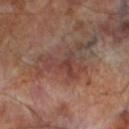No biopsy was performed on this lesion — it was imaged during a full skin examination and was not determined to be concerning. Captured under cross-polarized illumination. The patient is a male aged around 70. The lesion's longest dimension is about 4 mm. A roughly 15 mm field-of-view crop from a total-body skin photograph. The lesion is on the left lower leg. The total-body-photography lesion software estimated a mean CIELAB color near L≈39 a*≈21 b*≈22, roughly 7 lightness units darker than nearby skin, and a normalized border contrast of about 6. And it measured border irregularity of about 9 on a 0–10 scale, a color-variation rating of about 2.5/10, and peripheral color asymmetry of about 0.5. It also reported a nevus-likeness score of about 0/100 and a detector confidence of about 60 out of 100 that the crop contains a lesion.